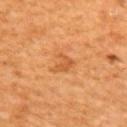follow-up=imaged on a skin check; not biopsied | lighting=cross-polarized | patient=male, approximately 60 years of age | imaging modality=15 mm crop, total-body photography | lesion diameter=about 2.5 mm | location=the upper back.